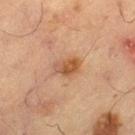Imaged during a routine full-body skin examination; the lesion was not biopsied and no histopathology is available. Automated tile analysis of the lesion measured an area of roughly 5.5 mm², an eccentricity of roughly 0.75, and a shape-asymmetry score of about 0.25 (0 = symmetric). The subject is a male approximately 65 years of age. The lesion is located on the right thigh. A 15 mm crop from a total-body photograph taken for skin-cancer surveillance. Longest diameter approximately 3 mm.